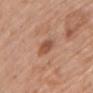{
  "biopsy_status": "not biopsied; imaged during a skin examination",
  "lighting": "white-light",
  "patient": {
    "sex": "female",
    "age_approx": 65
  },
  "site": "chest",
  "image": {
    "source": "total-body photography crop",
    "field_of_view_mm": 15
  }
}